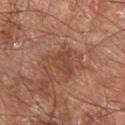A male patient about 60 years old.
Cropped from a total-body skin-imaging series; the visible field is about 15 mm.
The lesion's longest dimension is about 3.5 mm.
Located on the right leg.